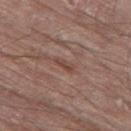| feature | finding |
|---|---|
| notes | imaged on a skin check; not biopsied |
| imaging modality | ~15 mm tile from a whole-body skin photo |
| tile lighting | white-light |
| image-analysis metrics | a border-irregularity rating of about 3.5/10, a color-variation rating of about 0/10, and a peripheral color-asymmetry measure near 0 |
| site | the left thigh |
| patient | male, approximately 80 years of age |